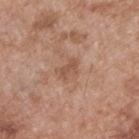biopsy status = no biopsy performed (imaged during a skin exam) | acquisition = 15 mm crop, total-body photography | lesion diameter = about 2.5 mm | subject = male, in their mid- to late 50s | lighting = white-light illumination | location = the upper back | TBP lesion metrics = internal color variation of about 1 on a 0–10 scale and radial color variation of about 0.5; a classifier nevus-likeness of about 0/100 and a detector confidence of about 100 out of 100 that the crop contains a lesion.